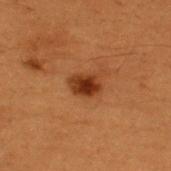Impression:
Part of a total-body skin-imaging series; this lesion was reviewed on a skin check and was not flagged for biopsy.
Context:
The lesion is located on the upper back. A 15 mm close-up extracted from a 3D total-body photography capture. Imaged with cross-polarized lighting. The lesion's longest dimension is about 3 mm. The patient is a male about 50 years old.